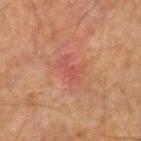Clinical impression: Recorded during total-body skin imaging; not selected for excision or biopsy. Background: A male subject, aged 63 to 67. The lesion is on the right forearm. A region of skin cropped from a whole-body photographic capture, roughly 15 mm wide.